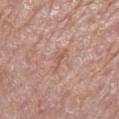follow-up=no biopsy performed (imaged during a skin exam) | anatomic site=the right lower leg | image=15 mm crop, total-body photography | subject=female, in their mid-70s | image-analysis metrics=an automated nevus-likeness rating near 0 out of 100 | lighting=white-light illumination | size=~2.5 mm (longest diameter).